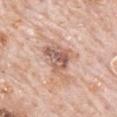Notes:
* notes — total-body-photography surveillance lesion; no biopsy